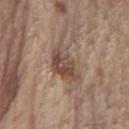Findings:
– biopsy status — total-body-photography surveillance lesion; no biopsy
– acquisition — 15 mm crop, total-body photography
– lighting — white-light illumination
– location — the left forearm
– patient — male, aged 68 to 72